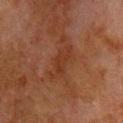Recorded during total-body skin imaging; not selected for excision or biopsy. The recorded lesion diameter is about 4.5 mm. A male subject aged 78–82. The lesion-visualizer software estimated a footprint of about 6 mm², a shape eccentricity near 0.9, and a symmetry-axis asymmetry near 0.3. The software also gave a lesion–skin lightness drop of about 5 and a normalized border contrast of about 5.5. And it measured border irregularity of about 4.5 on a 0–10 scale and radial color variation of about 0.5. The analysis additionally found a classifier nevus-likeness of about 0/100. A close-up tile cropped from a whole-body skin photograph, about 15 mm across. The tile uses cross-polarized illumination. The lesion is located on the chest.